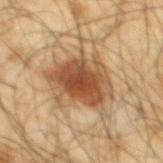follow-up = no biopsy performed (imaged during a skin exam); imaging modality = ~15 mm tile from a whole-body skin photo; lighting = cross-polarized; subject = male, aged 63 to 67; lesion diameter = ≈6 mm; site = the mid back.